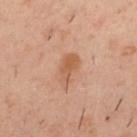biopsy status: total-body-photography surveillance lesion; no biopsy | anatomic site: the back | acquisition: ~15 mm crop, total-body skin-cancer survey | subject: male, roughly 40 years of age | illumination: cross-polarized illumination | lesion diameter: about 4 mm.